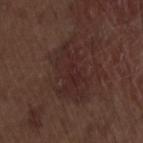| key | value |
|---|---|
| notes | total-body-photography surveillance lesion; no biopsy |
| patient | male, roughly 70 years of age |
| illumination | white-light illumination |
| acquisition | ~15 mm crop, total-body skin-cancer survey |
| lesion size | ~7 mm (longest diameter) |
| body site | the lower back |
| image-analysis metrics | a border-irregularity index near 4/10, a color-variation rating of about 3/10, and radial color variation of about 1; a classifier nevus-likeness of about 15/100 and lesion-presence confidence of about 95/100 |